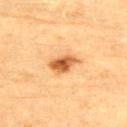Captured during whole-body skin photography for melanoma surveillance; the lesion was not biopsied. From the upper back. The lesion-visualizer software estimated a footprint of about 7 mm² and an eccentricity of roughly 0.8. And it measured an automated nevus-likeness rating near 95 out of 100 and a lesion-detection confidence of about 100/100. A male subject, in their mid- to late 80s or older. The tile uses cross-polarized illumination. A roughly 15 mm field-of-view crop from a total-body skin photograph.